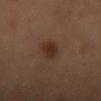A roughly 15 mm field-of-view crop from a total-body skin photograph. A female subject in their mid-50s. The lesion is located on the left forearm.